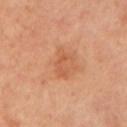biopsy status: imaged on a skin check; not biopsied
patient: female, approximately 60 years of age
body site: the arm
lesion diameter: ~3.5 mm (longest diameter)
image-analysis metrics: a lesion area of about 5.5 mm², a shape eccentricity near 0.8, and a symmetry-axis asymmetry near 0.4; a lesion color around L≈59 a*≈27 b*≈38 in CIELAB, a lesion–skin lightness drop of about 8, and a lesion-to-skin contrast of about 5.5 (normalized; higher = more distinct); a border-irregularity index near 5.5/10, internal color variation of about 2.5 on a 0–10 scale, and radial color variation of about 0.5; an automated nevus-likeness rating near 35 out of 100 and a detector confidence of about 100 out of 100 that the crop contains a lesion
image source: ~15 mm tile from a whole-body skin photo